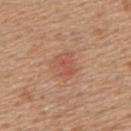Q: Was this lesion biopsied?
A: total-body-photography surveillance lesion; no biopsy
Q: How large is the lesion?
A: ≈3 mm
Q: Where on the body is the lesion?
A: the back
Q: Illumination type?
A: white-light illumination
Q: Automated lesion metrics?
A: a color-variation rating of about 2/10 and radial color variation of about 1
Q: What is the imaging modality?
A: total-body-photography crop, ~15 mm field of view
Q: What are the patient's age and sex?
A: female, aged approximately 55